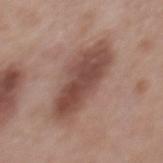patient = male, roughly 55 years of age | acquisition = 15 mm crop, total-body photography | lesion size = ~8.5 mm (longest diameter) | illumination = white-light | automated lesion analysis = a classifier nevus-likeness of about 85/100 and a lesion-detection confidence of about 100/100 | body site = the mid back.